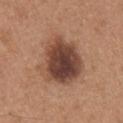Part of a total-body skin-imaging series; this lesion was reviewed on a skin check and was not flagged for biopsy. Located on the chest. The recorded lesion diameter is about 6 mm. The subject is a male aged 63 to 67. Cropped from a total-body skin-imaging series; the visible field is about 15 mm.